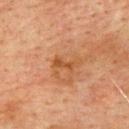Part of a total-body skin-imaging series; this lesion was reviewed on a skin check and was not flagged for biopsy. A region of skin cropped from a whole-body photographic capture, roughly 15 mm wide. An algorithmic analysis of the crop reported a footprint of about 3 mm², a shape eccentricity near 0.9, and a shape-asymmetry score of about 0.3 (0 = symmetric). And it measured a border-irregularity index near 3.5/10, a color-variation rating of about 0.5/10, and a peripheral color-asymmetry measure near 0. Located on the back. The patient is a male in their mid-70s. The tile uses cross-polarized illumination.